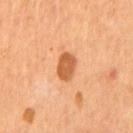Impression: Recorded during total-body skin imaging; not selected for excision or biopsy.